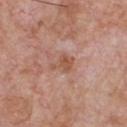Notes:
* follow-up: imaged on a skin check; not biopsied
* image source: total-body-photography crop, ~15 mm field of view
* subject: male, aged 58–62
* illumination: white-light
* automated lesion analysis: a lesion area of about 4 mm², an eccentricity of roughly 0.7, and a symmetry-axis asymmetry near 0.5; a mean CIELAB color near L≈53 a*≈22 b*≈30, a lesion–skin lightness drop of about 8, and a normalized lesion–skin contrast near 7; border irregularity of about 5 on a 0–10 scale and peripheral color asymmetry of about 0.5
* size: ≈3 mm
* body site: the chest